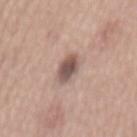Part of a total-body skin-imaging series; this lesion was reviewed on a skin check and was not flagged for biopsy.
A male patient, roughly 55 years of age.
From the mid back.
A 15 mm close-up extracted from a 3D total-body photography capture.